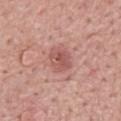This lesion was catalogued during total-body skin photography and was not selected for biopsy.
On the mid back.
Imaged with white-light lighting.
Cropped from a total-body skin-imaging series; the visible field is about 15 mm.
The recorded lesion diameter is about 3 mm.
The patient is a male in their 60s.